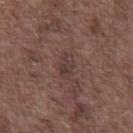biopsy status: no biopsy performed (imaged during a skin exam) | image-analysis metrics: a footprint of about 5 mm²; a mean CIELAB color near L≈37 a*≈16 b*≈18, roughly 7 lightness units darker than nearby skin, and a lesion-to-skin contrast of about 6.5 (normalized; higher = more distinct); a border-irregularity rating of about 2/10, internal color variation of about 4 on a 0–10 scale, and radial color variation of about 1.5; a classifier nevus-likeness of about 0/100 and a detector confidence of about 95 out of 100 that the crop contains a lesion | subject: male, approximately 70 years of age | lesion diameter: ~3 mm (longest diameter) | site: the abdomen | acquisition: total-body-photography crop, ~15 mm field of view | tile lighting: white-light.